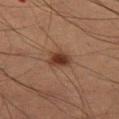Case summary:
– notes: imaged on a skin check; not biopsied
– imaging modality: ~15 mm crop, total-body skin-cancer survey
– body site: the leg
– patient: male, in their mid-50s